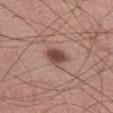Impression:
Part of a total-body skin-imaging series; this lesion was reviewed on a skin check and was not flagged for biopsy.
Acquisition and patient details:
Captured under white-light illumination. The lesion is located on the left thigh. The total-body-photography lesion software estimated a footprint of about 5 mm², an outline eccentricity of about 0.75 (0 = round, 1 = elongated), and a shape-asymmetry score of about 0.2 (0 = symmetric). It also reported lesion-presence confidence of about 100/100. This image is a 15 mm lesion crop taken from a total-body photograph. A male patient roughly 60 years of age. Longest diameter approximately 3 mm.